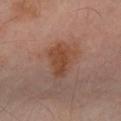Part of a total-body skin-imaging series; this lesion was reviewed on a skin check and was not flagged for biopsy. This is a cross-polarized tile. A male subject aged 53–57. Cropped from a total-body skin-imaging series; the visible field is about 15 mm. On the left forearm. The total-body-photography lesion software estimated about 8 CIELAB-L* units darker than the surrounding skin and a normalized lesion–skin contrast near 8. The software also gave a detector confidence of about 100 out of 100 that the crop contains a lesion. Approximately 4.5 mm at its widest.